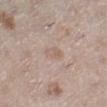A close-up tile cropped from a whole-body skin photograph, about 15 mm across. Automated tile analysis of the lesion measured about 6 CIELAB-L* units darker than the surrounding skin and a normalized lesion–skin contrast near 4.5. From the left lower leg. The patient is a female aged 38–42.